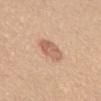This lesion was catalogued during total-body skin photography and was not selected for biopsy. Cropped from a total-body skin-imaging series; the visible field is about 15 mm. A female patient, aged approximately 55. From the chest. This is a white-light tile. About 3.5 mm across.